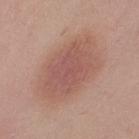No biopsy was performed on this lesion — it was imaged during a full skin examination and was not determined to be concerning. The subject is a female aged 28–32. The lesion's longest dimension is about 8 mm. Captured under white-light illumination. Cropped from a whole-body photographic skin survey; the tile spans about 15 mm. The lesion is on the right thigh.